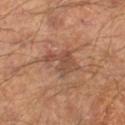Clinical impression:
Captured during whole-body skin photography for melanoma surveillance; the lesion was not biopsied.
Clinical summary:
Approximately 4.5 mm at its widest. The lesion is on the right lower leg. A lesion tile, about 15 mm wide, cut from a 3D total-body photograph. A male patient, aged 63–67.